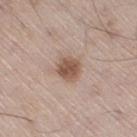Q: Was a biopsy performed?
A: imaged on a skin check; not biopsied
Q: Automated lesion metrics?
A: an area of roughly 6 mm², a shape eccentricity near 0.4, and a symmetry-axis asymmetry near 0.25; a border-irregularity index near 2/10, internal color variation of about 2.5 on a 0–10 scale, and peripheral color asymmetry of about 1; a nevus-likeness score of about 95/100 and a detector confidence of about 100 out of 100 that the crop contains a lesion
Q: Where on the body is the lesion?
A: the leg
Q: What lighting was used for the tile?
A: white-light
Q: Patient demographics?
A: male, approximately 55 years of age
Q: What is the lesion's diameter?
A: about 3 mm
Q: How was this image acquired?
A: total-body-photography crop, ~15 mm field of view